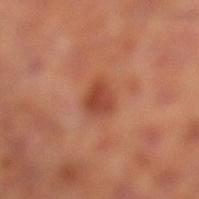Q: Was this lesion biopsied?
A: total-body-photography surveillance lesion; no biopsy